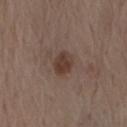Case summary:
• notes · no biopsy performed (imaged during a skin exam)
• size · ≈3 mm
• anatomic site · the mid back
• imaging modality · total-body-photography crop, ~15 mm field of view
• patient · male, aged around 70
• tile lighting · white-light